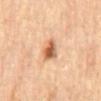{
  "biopsy_status": "not biopsied; imaged during a skin examination",
  "automated_metrics": {
    "area_mm2_approx": 5.5,
    "eccentricity": 0.55,
    "shape_asymmetry": 0.25,
    "cielab_L": 60,
    "cielab_a": 24,
    "cielab_b": 37,
    "vs_skin_darker_L": 15.0,
    "vs_skin_contrast_norm": 9.5,
    "border_irregularity_0_10": 2.5,
    "color_variation_0_10": 8.5,
    "peripheral_color_asymmetry": 3.0,
    "nevus_likeness_0_100": 95
  },
  "lighting": "cross-polarized",
  "site": "mid back",
  "patient": {
    "sex": "male",
    "age_approx": "85+"
  },
  "image": {
    "source": "total-body photography crop",
    "field_of_view_mm": 15
  },
  "lesion_size": {
    "long_diameter_mm_approx": 3.0
  }
}